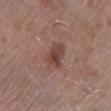{"biopsy_status": "not biopsied; imaged during a skin examination", "patient": {"sex": "female", "age_approx": "85+"}, "lesion_size": {"long_diameter_mm_approx": 3.5}, "lighting": "white-light", "automated_metrics": {"area_mm2_approx": 7.0, "eccentricity": 0.7, "shape_asymmetry": 0.25, "cielab_L": 43, "cielab_a": 20, "cielab_b": 23, "vs_skin_darker_L": 9.0, "vs_skin_contrast_norm": 7.5, "lesion_detection_confidence_0_100": 100}, "site": "left lower leg", "image": {"source": "total-body photography crop", "field_of_view_mm": 15}}